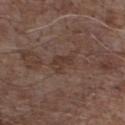Impression:
The lesion was tiled from a total-body skin photograph and was not biopsied.
Clinical summary:
The lesion is on the chest. A male patient aged 68–72. Automated tile analysis of the lesion measured an area of roughly 4 mm², a shape eccentricity near 0.85, and a symmetry-axis asymmetry near 0.45. Cropped from a whole-body photographic skin survey; the tile spans about 15 mm. Captured under white-light illumination.